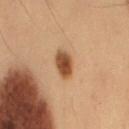This lesion was catalogued during total-body skin photography and was not selected for biopsy.
The tile uses cross-polarized illumination.
About 3 mm across.
A male patient, aged 53–57.
On the lower back.
A roughly 15 mm field-of-view crop from a total-body skin photograph.